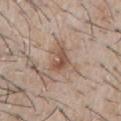Clinical impression:
The lesion was photographed on a routine skin check and not biopsied; there is no pathology result.
Context:
This is a white-light tile. A male patient roughly 65 years of age. Located on the chest. About 3.5 mm across. A 15 mm crop from a total-body photograph taken for skin-cancer surveillance.